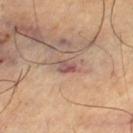A 15 mm crop from a total-body photograph taken for skin-cancer surveillance. Measured at roughly 2.5 mm in maximum diameter. Automated tile analysis of the lesion measured a mean CIELAB color near L≈52 a*≈21 b*≈23, roughly 10 lightness units darker than nearby skin, and a normalized lesion–skin contrast near 8. And it measured a border-irregularity index near 3.5/10, a color-variation rating of about 6/10, and radial color variation of about 2.5. And it measured a nevus-likeness score of about 0/100 and a detector confidence of about 75 out of 100 that the crop contains a lesion. A male subject aged approximately 65. This is a cross-polarized tile. On the left thigh.